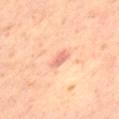The lesion-visualizer software estimated a mean CIELAB color near L≈68 a*≈27 b*≈31. It also reported a border-irregularity rating of about 2.5/10, a color-variation rating of about 1/10, and radial color variation of about 0. It also reported a nevus-likeness score of about 10/100 and lesion-presence confidence of about 100/100.
The lesion is located on the left thigh.
Captured under cross-polarized illumination.
A region of skin cropped from a whole-body photographic capture, roughly 15 mm wide.
A male subject in their mid-60s.